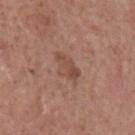Assessment: Captured during whole-body skin photography for melanoma surveillance; the lesion was not biopsied. Context: The lesion is on the head or neck. Cropped from a total-body skin-imaging series; the visible field is about 15 mm. Automated tile analysis of the lesion measured an area of roughly 7 mm². The analysis additionally found a mean CIELAB color near L≈48 a*≈21 b*≈26, roughly 7 lightness units darker than nearby skin, and a normalized lesion–skin contrast near 5.5. The analysis additionally found border irregularity of about 3 on a 0–10 scale and internal color variation of about 3 on a 0–10 scale. And it measured a lesion-detection confidence of about 100/100. The patient is a male approximately 70 years of age. This is a white-light tile.